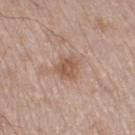biopsy status — total-body-photography surveillance lesion; no biopsy
acquisition — ~15 mm tile from a whole-body skin photo
diameter — ≈3 mm
patient — male, aged 53 to 57
illumination — white-light
anatomic site — the right thigh
image-analysis metrics — an area of roughly 5.5 mm² and a shape eccentricity near 0.45; a lesion color around L≈55 a*≈19 b*≈28 in CIELAB, about 9 CIELAB-L* units darker than the surrounding skin, and a normalized border contrast of about 7